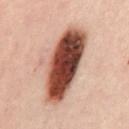This lesion was catalogued during total-body skin photography and was not selected for biopsy.
Imaged with cross-polarized lighting.
A female subject, about 40 years old.
The recorded lesion diameter is about 9.5 mm.
The total-body-photography lesion software estimated a border-irregularity rating of about 3/10, a color-variation rating of about 10/10, and peripheral color asymmetry of about 3.
From the mid back.
This image is a 15 mm lesion crop taken from a total-body photograph.